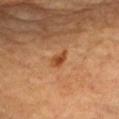Clinical impression: Recorded during total-body skin imaging; not selected for excision or biopsy. Background: Captured under cross-polarized illumination. A lesion tile, about 15 mm wide, cut from a 3D total-body photograph. A male subject, roughly 85 years of age or older. From the chest. Measured at roughly 2.5 mm in maximum diameter.